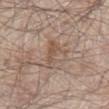Q: Is there a histopathology result?
A: imaged on a skin check; not biopsied
Q: Illumination type?
A: white-light
Q: What are the patient's age and sex?
A: male, aged approximately 80
Q: Where on the body is the lesion?
A: the abdomen
Q: What is the lesion's diameter?
A: ≈4.5 mm
Q: Automated lesion metrics?
A: a mean CIELAB color near L≈54 a*≈16 b*≈26, a lesion–skin lightness drop of about 7, and a lesion-to-skin contrast of about 5 (normalized; higher = more distinct); an automated nevus-likeness rating near 0 out of 100 and a detector confidence of about 95 out of 100 that the crop contains a lesion
Q: What is the imaging modality?
A: total-body-photography crop, ~15 mm field of view